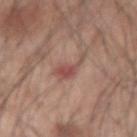{
  "biopsy_status": "not biopsied; imaged during a skin examination",
  "image": {
    "source": "total-body photography crop",
    "field_of_view_mm": 15
  },
  "patient": {
    "sex": "male",
    "age_approx": 45
  },
  "site": "right forearm"
}